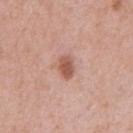Part of a total-body skin-imaging series; this lesion was reviewed on a skin check and was not flagged for biopsy. A male subject, aged 38 to 42. Captured under white-light illumination. The lesion is on the abdomen. Longest diameter approximately 3 mm. The total-body-photography lesion software estimated a shape eccentricity near 0.65 and a symmetry-axis asymmetry near 0.2. It also reported a mean CIELAB color near L≈56 a*≈23 b*≈28, about 12 CIELAB-L* units darker than the surrounding skin, and a normalized lesion–skin contrast near 8. The software also gave border irregularity of about 2 on a 0–10 scale and a color-variation rating of about 2.5/10. The analysis additionally found an automated nevus-likeness rating near 95 out of 100 and lesion-presence confidence of about 100/100. A 15 mm close-up tile from a total-body photography series done for melanoma screening.